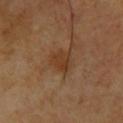No biopsy was performed on this lesion — it was imaged during a full skin examination and was not determined to be concerning. The patient is a male aged around 65. The lesion is on the upper back. This image is a 15 mm lesion crop taken from a total-body photograph.